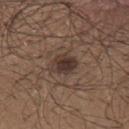The lesion was photographed on a routine skin check and not biopsied; there is no pathology result.
A close-up tile cropped from a whole-body skin photograph, about 15 mm across.
Longest diameter approximately 3.5 mm.
The lesion is on the upper back.
A male subject, roughly 25 years of age.